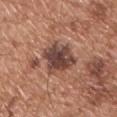Captured during whole-body skin photography for melanoma surveillance; the lesion was not biopsied. A male patient, in their mid-50s. The lesion-visualizer software estimated a normalized border contrast of about 10.5. It also reported a nevus-likeness score of about 15/100 and lesion-presence confidence of about 100/100. Captured under white-light illumination. The lesion is located on the chest. About 5 mm across. A 15 mm close-up extracted from a 3D total-body photography capture.